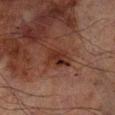{
  "biopsy_status": "not biopsied; imaged during a skin examination",
  "lesion_size": {
    "long_diameter_mm_approx": 4.0
  },
  "automated_metrics": {
    "area_mm2_approx": 7.5,
    "eccentricity": 0.8,
    "shape_asymmetry": 0.2,
    "cielab_L": 25,
    "cielab_a": 19,
    "cielab_b": 22,
    "vs_skin_darker_L": 7.0,
    "vs_skin_contrast_norm": 8.0
  },
  "site": "right lower leg",
  "patient": {
    "sex": "male",
    "age_approx": 70
  },
  "image": {
    "source": "total-body photography crop",
    "field_of_view_mm": 15
  }
}